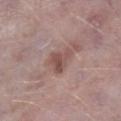notes: imaged on a skin check; not biopsied | lighting: white-light illumination | automated metrics: a mean CIELAB color near L≈50 a*≈19 b*≈22 and a lesion–skin lightness drop of about 9 | lesion size: ~3 mm (longest diameter) | patient: male, aged approximately 75 | acquisition: total-body-photography crop, ~15 mm field of view | location: the right lower leg.